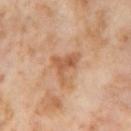workup: catalogued during a skin exam; not biopsied
location: the right thigh
lesion diameter: about 4 mm
tile lighting: cross-polarized illumination
image-analysis metrics: a border-irregularity index near 7/10, a color-variation rating of about 3/10, and peripheral color asymmetry of about 1
acquisition: ~15 mm tile from a whole-body skin photo
patient: female, aged 53 to 57